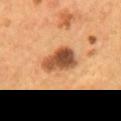Impression:
This lesion was catalogued during total-body skin photography and was not selected for biopsy.
Background:
The lesion is on the back. An algorithmic analysis of the crop reported a lesion area of about 12 mm² and a symmetry-axis asymmetry near 0.25. And it measured an average lesion color of about L≈48 a*≈22 b*≈35 (CIELAB) and a normalized border contrast of about 11. It also reported a border-irregularity rating of about 2.5/10, a color-variation rating of about 8/10, and radial color variation of about 2.5. The patient is a male approximately 55 years of age. This image is a 15 mm lesion crop taken from a total-body photograph. The lesion's longest dimension is about 4.5 mm. Imaged with cross-polarized lighting.